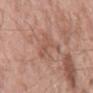{"biopsy_status": "not biopsied; imaged during a skin examination", "lighting": "white-light", "site": "back", "automated_metrics": {"area_mm2_approx": 6.5, "cielab_L": 54, "cielab_a": 21, "cielab_b": 28, "vs_skin_darker_L": 7.0, "vs_skin_contrast_norm": 5.0, "border_irregularity_0_10": 8.0, "color_variation_0_10": 2.0, "peripheral_color_asymmetry": 0.5, "nevus_likeness_0_100": 0, "lesion_detection_confidence_0_100": 100}, "patient": {"sex": "male", "age_approx": 60}, "image": {"source": "total-body photography crop", "field_of_view_mm": 15}, "lesion_size": {"long_diameter_mm_approx": 5.0}}